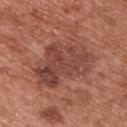A 15 mm close-up extracted from a 3D total-body photography capture.
A female patient, aged 38 to 42.
The lesion is on the upper back.
Automated image analysis of the tile measured a lesion area of about 27 mm² and a shape-asymmetry score of about 0.35 (0 = symmetric). And it measured a mean CIELAB color near L≈44 a*≈25 b*≈25, roughly 9 lightness units darker than nearby skin, and a lesion-to-skin contrast of about 7.5 (normalized; higher = more distinct). The analysis additionally found a within-lesion color-variation index near 5.5/10 and peripheral color asymmetry of about 2. And it measured a nevus-likeness score of about 0/100 and a detector confidence of about 100 out of 100 that the crop contains a lesion.